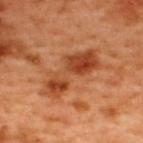Assessment:
This lesion was catalogued during total-body skin photography and was not selected for biopsy.
Clinical summary:
The lesion is located on the upper back. Measured at roughly 7 mm in maximum diameter. The subject is a male aged 48 to 52. Automated image analysis of the tile measured a lesion area of about 16 mm², a shape eccentricity near 0.9, and a symmetry-axis asymmetry near 0.4. The software also gave border irregularity of about 5.5 on a 0–10 scale. The analysis additionally found a nevus-likeness score of about 20/100 and a detector confidence of about 100 out of 100 that the crop contains a lesion. Imaged with cross-polarized lighting. A close-up tile cropped from a whole-body skin photograph, about 15 mm across.